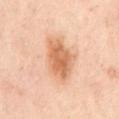{
  "biopsy_status": "not biopsied; imaged during a skin examination",
  "image": {
    "source": "total-body photography crop",
    "field_of_view_mm": 15
  },
  "lesion_size": {
    "long_diameter_mm_approx": 6.0
  },
  "site": "mid back",
  "lighting": "cross-polarized",
  "patient": {
    "sex": "female",
    "age_approx": 60
  }
}